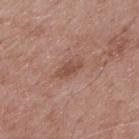Findings:
• workup: imaged on a skin check; not biopsied
• tile lighting: white-light
• automated metrics: a normalized border contrast of about 7
• diameter: ~3 mm (longest diameter)
• anatomic site: the upper back
• subject: male, about 70 years old
• image source: total-body-photography crop, ~15 mm field of view